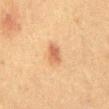acquisition: 15 mm crop, total-body photography
patient: male, aged around 50
location: the front of the torso